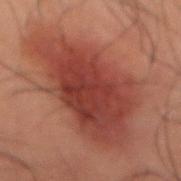workup: catalogued during a skin exam; not biopsied
subject: male, aged approximately 50
lesion size: ~11.5 mm (longest diameter)
image source: total-body-photography crop, ~15 mm field of view
automated lesion analysis: an area of roughly 55 mm² and an eccentricity of roughly 0.8; about 9 CIELAB-L* units darker than the surrounding skin; border irregularity of about 4 on a 0–10 scale and a color-variation rating of about 4/10; a nevus-likeness score of about 100/100 and a lesion-detection confidence of about 100/100
body site: the abdomen